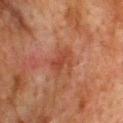The lesion was photographed on a routine skin check and not biopsied; there is no pathology result.
The lesion is on the upper back.
The subject is a male in their mid-70s.
A close-up tile cropped from a whole-body skin photograph, about 15 mm across.